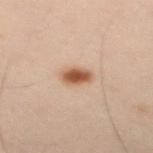Part of a total-body skin-imaging series; this lesion was reviewed on a skin check and was not flagged for biopsy. The recorded lesion diameter is about 2.5 mm. A male patient, aged 48–52. The tile uses cross-polarized illumination. Cropped from a whole-body photographic skin survey; the tile spans about 15 mm. On the left arm.